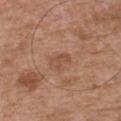Assessment: Imaged during a routine full-body skin examination; the lesion was not biopsied and no histopathology is available. Background: A close-up tile cropped from a whole-body skin photograph, about 15 mm across. Automated image analysis of the tile measured a footprint of about 3.5 mm², an outline eccentricity of about 0.8 (0 = round, 1 = elongated), and a symmetry-axis asymmetry near 0.35. And it measured an average lesion color of about L≈50 a*≈22 b*≈30 (CIELAB), roughly 7 lightness units darker than nearby skin, and a normalized lesion–skin contrast near 5.5. A male subject, roughly 75 years of age. The tile uses white-light illumination. Measured at roughly 2.5 mm in maximum diameter. The lesion is located on the front of the torso.